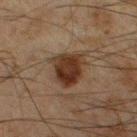Impression:
Imaged during a routine full-body skin examination; the lesion was not biopsied and no histopathology is available.
Background:
From the left lower leg. A roughly 15 mm field-of-view crop from a total-body skin photograph. The tile uses cross-polarized illumination. A male patient aged around 45.